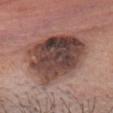Assessment: Part of a total-body skin-imaging series; this lesion was reviewed on a skin check and was not flagged for biopsy. Acquisition and patient details: The lesion is located on the head or neck. The subject is a female approximately 60 years of age. Cropped from a total-body skin-imaging series; the visible field is about 15 mm. The tile uses white-light illumination. Measured at roughly 8 mm in maximum diameter.